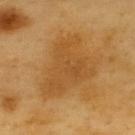patient = male, aged 58 to 62 | anatomic site = the upper back | illumination = cross-polarized | image source = 15 mm crop, total-body photography.